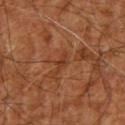biopsy status — imaged on a skin check; not biopsied
subject — approximately 65 years of age
image-analysis metrics — an area of roughly 3 mm², an outline eccentricity of about 0.85 (0 = round, 1 = elongated), and a shape-asymmetry score of about 0.4 (0 = symmetric); a border-irregularity index near 4.5/10 and a peripheral color-asymmetry measure near 1
illumination — cross-polarized illumination
acquisition — total-body-photography crop, ~15 mm field of view
anatomic site — the right upper arm